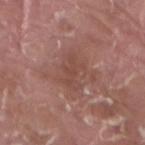follow-up: catalogued during a skin exam; not biopsied | patient: male, about 40 years old | illumination: white-light illumination | site: the right thigh | image-analysis metrics: two-axis asymmetry of about 0.5; a border-irregularity index near 7/10 and peripheral color asymmetry of about 0.5 | imaging modality: ~15 mm crop, total-body skin-cancer survey.